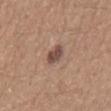Captured during whole-body skin photography for melanoma surveillance; the lesion was not biopsied.
A male subject, about 20 years old.
The lesion is on the mid back.
Cropped from a total-body skin-imaging series; the visible field is about 15 mm.